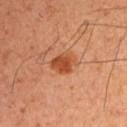lesion_size:
  long_diameter_mm_approx: 3.0
patient:
  sex: male
  age_approx: 50
site: left upper arm
lighting: cross-polarized
image:
  source: total-body photography crop
  field_of_view_mm: 15
automated_metrics:
  eccentricity: 0.6
  shape_asymmetry: 0.2
  cielab_L: 47
  cielab_a: 28
  cielab_b: 37
  vs_skin_contrast_norm: 8.5
  border_irregularity_0_10: 2.0
  color_variation_0_10: 2.5